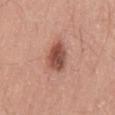Image and clinical context: The tile uses white-light illumination. A male patient, about 40 years old. Located on the lower back. A close-up tile cropped from a whole-body skin photograph, about 15 mm across. The total-body-photography lesion software estimated an average lesion color of about L≈50 a*≈25 b*≈28 (CIELAB), a lesion–skin lightness drop of about 14, and a normalized border contrast of about 9.5. It also reported a border-irregularity index near 2/10, a within-lesion color-variation index near 5/10, and peripheral color asymmetry of about 1.5.